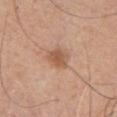{"biopsy_status": "not biopsied; imaged during a skin examination", "automated_metrics": {"area_mm2_approx": 6.0, "eccentricity": 0.7, "border_irregularity_0_10": 2.5, "peripheral_color_asymmetry": 1.0, "nevus_likeness_0_100": 30, "lesion_detection_confidence_0_100": 100}, "site": "front of the torso", "patient": {"sex": "male", "age_approx": 65}, "lesion_size": {"long_diameter_mm_approx": 3.0}, "image": {"source": "total-body photography crop", "field_of_view_mm": 15}}